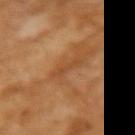workup: no biopsy performed (imaged during a skin exam) | TBP lesion metrics: an area of roughly 3 mm², an outline eccentricity of about 0.95 (0 = round, 1 = elongated), and a shape-asymmetry score of about 0.35 (0 = symmetric); a border-irregularity rating of about 4/10, internal color variation of about 0 on a 0–10 scale, and radial color variation of about 0 | illumination: cross-polarized | size: ≈3 mm | subject: female, aged around 70 | anatomic site: the right forearm | image: 15 mm crop, total-body photography.